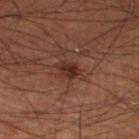Assessment:
The lesion was tiled from a total-body skin photograph and was not biopsied.
Image and clinical context:
Automated image analysis of the tile measured an average lesion color of about L≈26 a*≈20 b*≈23 (CIELAB), roughly 9 lightness units darker than nearby skin, and a normalized lesion–skin contrast near 9.5. Captured under cross-polarized illumination. A male patient, approximately 60 years of age. Located on the left lower leg. Cropped from a whole-body photographic skin survey; the tile spans about 15 mm.